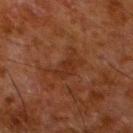No biopsy was performed on this lesion — it was imaged during a full skin examination and was not determined to be concerning. A close-up tile cropped from a whole-body skin photograph, about 15 mm across. This is a cross-polarized tile. Measured at roughly 5 mm in maximum diameter. A male subject aged 78–82. On the left lower leg.